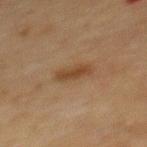{
  "biopsy_status": "not biopsied; imaged during a skin examination"
}